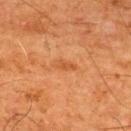Case summary:
• biopsy status — imaged on a skin check; not biopsied
• patient — male, about 65 years old
• acquisition — total-body-photography crop, ~15 mm field of view
• body site — the upper back
• lesion size — about 2.5 mm